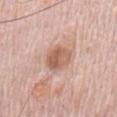– biopsy status: imaged on a skin check; not biopsied
– lesion diameter: ~3.5 mm (longest diameter)
– subject: male, about 70 years old
– site: the chest
– image: ~15 mm tile from a whole-body skin photo
– illumination: white-light illumination